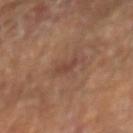Clinical impression: No biopsy was performed on this lesion — it was imaged during a full skin examination and was not determined to be concerning. Clinical summary: The subject is a male aged 63 to 67. A 15 mm close-up extracted from a 3D total-body photography capture. Located on the right forearm. Captured under cross-polarized illumination. Longest diameter approximately 2.5 mm.